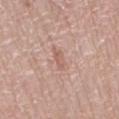This lesion was catalogued during total-body skin photography and was not selected for biopsy. This is a white-light tile. A male subject, aged approximately 75. On the leg. An algorithmic analysis of the crop reported a lesion area of about 3 mm², an outline eccentricity of about 0.9 (0 = round, 1 = elongated), and a symmetry-axis asymmetry near 0.35. And it measured a lesion–skin lightness drop of about 8 and a lesion-to-skin contrast of about 5 (normalized; higher = more distinct). The software also gave an automated nevus-likeness rating near 0 out of 100 and lesion-presence confidence of about 100/100. Cropped from a total-body skin-imaging series; the visible field is about 15 mm. Longest diameter approximately 3 mm.